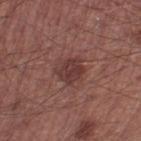notes: imaged on a skin check; not biopsied | subject: male, aged around 45 | anatomic site: the leg | diameter: about 3.5 mm | image: ~15 mm crop, total-body skin-cancer survey | TBP lesion metrics: a normalized border contrast of about 7; a nevus-likeness score of about 45/100.